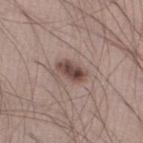workup: imaged on a skin check; not biopsied
image: ~15 mm crop, total-body skin-cancer survey
tile lighting: white-light
lesion size: about 3.5 mm
TBP lesion metrics: a footprint of about 6 mm² and a shape eccentricity near 0.85; an average lesion color of about L≈46 a*≈17 b*≈21 (CIELAB), about 13 CIELAB-L* units darker than the surrounding skin, and a lesion-to-skin contrast of about 10 (normalized; higher = more distinct); border irregularity of about 2 on a 0–10 scale, a color-variation rating of about 5/10, and peripheral color asymmetry of about 2
anatomic site: the left thigh
patient: male, aged 23 to 27